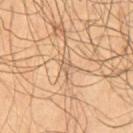{"biopsy_status": "not biopsied; imaged during a skin examination", "lighting": "cross-polarized", "patient": {"sex": "male", "age_approx": 65}, "image": {"source": "total-body photography crop", "field_of_view_mm": 15}, "lesion_size": {"long_diameter_mm_approx": 1.5}, "automated_metrics": {"area_mm2_approx": 1.5, "eccentricity": 0.65, "shape_asymmetry": 0.3, "cielab_L": 60, "cielab_a": 16, "cielab_b": 33, "vs_skin_darker_L": 7.0, "vs_skin_contrast_norm": 4.5}, "site": "chest"}